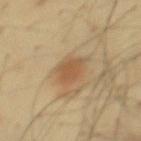No biopsy was performed on this lesion — it was imaged during a full skin examination and was not determined to be concerning.
An algorithmic analysis of the crop reported a lesion color around L≈52 a*≈18 b*≈34 in CIELAB and a lesion–skin lightness drop of about 9. The analysis additionally found a within-lesion color-variation index near 2/10 and peripheral color asymmetry of about 0.5. And it measured a classifier nevus-likeness of about 95/100 and lesion-presence confidence of about 100/100.
The lesion's longest dimension is about 3 mm.
The tile uses cross-polarized illumination.
A 15 mm crop from a total-body photograph taken for skin-cancer surveillance.
The lesion is on the mid back.
A male subject aged 43 to 47.